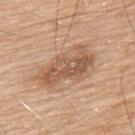No biopsy was performed on this lesion — it was imaged during a full skin examination and was not determined to be concerning. Located on the upper back. A male subject approximately 80 years of age. Longest diameter approximately 7 mm. A 15 mm close-up tile from a total-body photography series done for melanoma screening. Captured under white-light illumination.